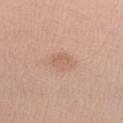Recorded during total-body skin imaging; not selected for excision or biopsy.
The lesion is on the left forearm.
Cropped from a whole-body photographic skin survey; the tile spans about 15 mm.
A female patient approximately 40 years of age.